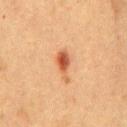Impression: Part of a total-body skin-imaging series; this lesion was reviewed on a skin check and was not flagged for biopsy. Context: A female subject aged around 40. Automated tile analysis of the lesion measured a within-lesion color-variation index near 5/10 and radial color variation of about 1.5. A 15 mm close-up extracted from a 3D total-body photography capture. Captured under cross-polarized illumination. Located on the abdomen. Longest diameter approximately 4 mm.